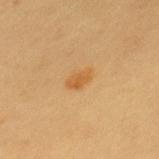Assessment:
Captured during whole-body skin photography for melanoma surveillance; the lesion was not biopsied.
Acquisition and patient details:
Located on the mid back. A 15 mm close-up extracted from a 3D total-body photography capture. This is a cross-polarized tile. A female patient, about 20 years old.